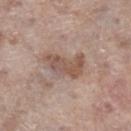- notes · catalogued during a skin exam; not biopsied
- lesion size · ~5 mm (longest diameter)
- site · the right lower leg
- lighting · white-light illumination
- subject · female, aged 83 or older
- acquisition · total-body-photography crop, ~15 mm field of view